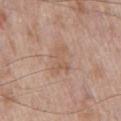The lesion was tiled from a total-body skin photograph and was not biopsied.